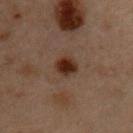Clinical summary: Longest diameter approximately 2.5 mm. A male subject, roughly 55 years of age. The tile uses cross-polarized illumination. On the front of the torso. Cropped from a whole-body photographic skin survey; the tile spans about 15 mm.